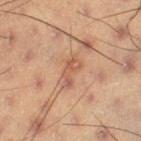Part of a total-body skin-imaging series; this lesion was reviewed on a skin check and was not flagged for biopsy.